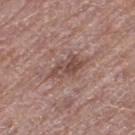biopsy_status: not biopsied; imaged during a skin examination
lighting: white-light
image:
  source: total-body photography crop
  field_of_view_mm: 15
site: left thigh
automated_metrics:
  cielab_L: 47
  cielab_a: 19
  cielab_b: 23
  vs_skin_darker_L: 10.0
  vs_skin_contrast_norm: 7.5
  border_irregularity_0_10: 4.0
  color_variation_0_10: 4.0
  peripheral_color_asymmetry: 1.5
lesion_size:
  long_diameter_mm_approx: 4.0
patient:
  sex: male
  age_approx: 65